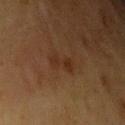The lesion was photographed on a routine skin check and not biopsied; there is no pathology result.
The tile uses cross-polarized illumination.
Located on the left upper arm.
Longest diameter approximately 3.5 mm.
The patient is a female roughly 55 years of age.
A 15 mm crop from a total-body photograph taken for skin-cancer surveillance.